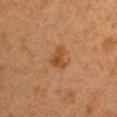The lesion was photographed on a routine skin check and not biopsied; there is no pathology result. About 3 mm across. Imaged with cross-polarized lighting. Cropped from a total-body skin-imaging series; the visible field is about 15 mm. The lesion is on the right upper arm. A female patient roughly 40 years of age.